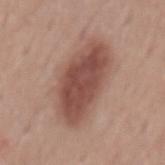follow-up = no biopsy performed (imaged during a skin exam)
image = ~15 mm crop, total-body skin-cancer survey
illumination = white-light
lesion diameter = ~8.5 mm (longest diameter)
body site = the mid back
automated metrics = a mean CIELAB color near L≈48 a*≈22 b*≈25, a lesion–skin lightness drop of about 14, and a normalized lesion–skin contrast near 9.5
subject = male, aged approximately 55